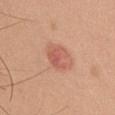biopsy status = total-body-photography surveillance lesion; no biopsy
image = 15 mm crop, total-body photography
location = the chest
subject = male, aged 38 to 42